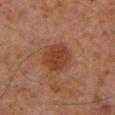<case>
  <biopsy_status>not biopsied; imaged during a skin examination</biopsy_status>
</case>